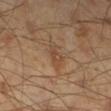biopsy_status: not biopsied; imaged during a skin examination
patient:
  sex: male
  age_approx: 45
automated_metrics:
  vs_skin_darker_L: 6.0
  vs_skin_contrast_norm: 5.5
image:
  source: total-body photography crop
  field_of_view_mm: 15
lesion_size:
  long_diameter_mm_approx: 3.0
site: leg
lighting: cross-polarized